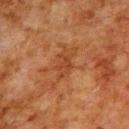notes: catalogued during a skin exam; not biopsied | lesion diameter: ≈3.5 mm | tile lighting: cross-polarized illumination | automated lesion analysis: a mean CIELAB color near L≈34 a*≈23 b*≈31, about 6 CIELAB-L* units darker than the surrounding skin, and a normalized border contrast of about 5.5 | acquisition: ~15 mm tile from a whole-body skin photo | location: the upper back | patient: male, aged approximately 80.